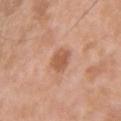This lesion was catalogued during total-body skin photography and was not selected for biopsy. Captured under white-light illumination. A close-up tile cropped from a whole-body skin photograph, about 15 mm across. The lesion's longest dimension is about 3 mm. A male subject in their mid-30s. From the front of the torso.